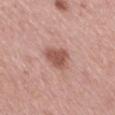follow-up: catalogued during a skin exam; not biopsied
size: ≈3 mm
site: the right thigh
image source: total-body-photography crop, ~15 mm field of view
subject: female, aged approximately 55
TBP lesion metrics: an automated nevus-likeness rating near 75 out of 100